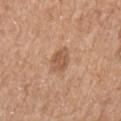Case summary:
– follow-up — imaged on a skin check; not biopsied
– lesion diameter — about 3 mm
– subject — female, aged approximately 70
– image — total-body-photography crop, ~15 mm field of view
– site — the left upper arm
– automated lesion analysis — an eccentricity of roughly 0.55 and two-axis asymmetry of about 0.2; an average lesion color of about L≈55 a*≈21 b*≈32 (CIELAB) and a lesion–skin lightness drop of about 10; a classifier nevus-likeness of about 5/100 and a detector confidence of about 100 out of 100 that the crop contains a lesion